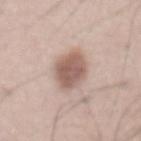workup = imaged on a skin check; not biopsied
imaging modality = 15 mm crop, total-body photography
lesion diameter = about 5 mm
subject = male, aged 53 to 57
tile lighting = white-light illumination